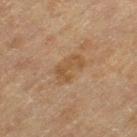Assessment:
This lesion was catalogued during total-body skin photography and was not selected for biopsy.
Acquisition and patient details:
The patient is a female about 55 years old. A lesion tile, about 15 mm wide, cut from a 3D total-body photograph. The tile uses cross-polarized illumination. The lesion's longest dimension is about 4 mm. The lesion is on the left thigh. The lesion-visualizer software estimated border irregularity of about 3.5 on a 0–10 scale, a within-lesion color-variation index near 2/10, and radial color variation of about 1.